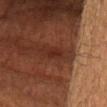Recorded during total-body skin imaging; not selected for excision or biopsy. The lesion's longest dimension is about 2.5 mm. Located on the head or neck. The subject is a male approximately 65 years of age. This image is a 15 mm lesion crop taken from a total-body photograph. Automated image analysis of the tile measured an area of roughly 3 mm², an outline eccentricity of about 0.8 (0 = round, 1 = elongated), and a shape-asymmetry score of about 0.3 (0 = symmetric). It also reported a mean CIELAB color near L≈22 a*≈20 b*≈23, a lesion–skin lightness drop of about 5, and a normalized border contrast of about 6.5. It also reported a border-irregularity rating of about 3/10 and radial color variation of about 0.5. And it measured a classifier nevus-likeness of about 0/100 and a detector confidence of about 90 out of 100 that the crop contains a lesion. Captured under cross-polarized illumination.